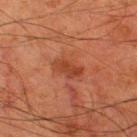No biopsy was performed on this lesion — it was imaged during a full skin examination and was not determined to be concerning. The lesion is on the right thigh. A male patient, aged around 80. Cropped from a whole-body photographic skin survey; the tile spans about 15 mm. Measured at roughly 3.5 mm in maximum diameter.